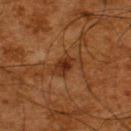The lesion was tiled from a total-body skin photograph and was not biopsied.
The lesion's longest dimension is about 4 mm.
The lesion-visualizer software estimated an outline eccentricity of about 0.85 (0 = round, 1 = elongated) and a symmetry-axis asymmetry near 0.4. It also reported an average lesion color of about L≈27 a*≈19 b*≈28 (CIELAB), roughly 7 lightness units darker than nearby skin, and a normalized lesion–skin contrast near 7.5. The software also gave a border-irregularity rating of about 5/10, a color-variation rating of about 3.5/10, and peripheral color asymmetry of about 1. And it measured an automated nevus-likeness rating near 55 out of 100 and a lesion-detection confidence of about 100/100.
Imaged with cross-polarized lighting.
Located on the upper back.
The patient is a male aged around 65.
Cropped from a whole-body photographic skin survey; the tile spans about 15 mm.